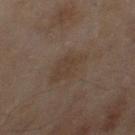follow-up: catalogued during a skin exam; not biopsied | illumination: cross-polarized | lesion diameter: ~4.5 mm (longest diameter) | anatomic site: the right thigh | subject: female, approximately 60 years of age | image: ~15 mm crop, total-body skin-cancer survey.